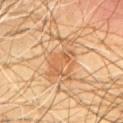Notes:
* biopsy status: catalogued during a skin exam; not biopsied
* subject: male, aged 58–62
* image: total-body-photography crop, ~15 mm field of view
* lesion size: about 5.5 mm
* anatomic site: the chest